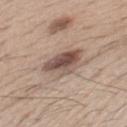{"biopsy_status": "not biopsied; imaged during a skin examination", "automated_metrics": {"eccentricity": 0.8, "shape_asymmetry": 0.2, "cielab_L": 52, "cielab_a": 16, "cielab_b": 23, "vs_skin_darker_L": 13.0}, "image": {"source": "total-body photography crop", "field_of_view_mm": 15}, "lesion_size": {"long_diameter_mm_approx": 5.5}, "lighting": "white-light", "site": "mid back", "patient": {"sex": "male", "age_approx": 40}}